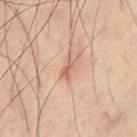<lesion>
<image>
  <source>total-body photography crop</source>
  <field_of_view_mm>15</field_of_view_mm>
</image>
<patient>
  <sex>male</sex>
  <age_approx>60</age_approx>
</patient>
<site>chest</site>
<lesion_size>
  <long_diameter_mm_approx>3.0</long_diameter_mm_approx>
</lesion_size>
</lesion>